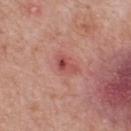Q: Was this lesion biopsied?
A: total-body-photography surveillance lesion; no biopsy
Q: Lesion location?
A: the back
Q: What is the imaging modality?
A: ~15 mm tile from a whole-body skin photo
Q: Who is the patient?
A: male, in their mid-60s
Q: What is the lesion's diameter?
A: about 3 mm
Q: Illumination type?
A: white-light